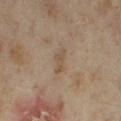Q: Was a biopsy performed?
A: no biopsy performed (imaged during a skin exam)
Q: Where on the body is the lesion?
A: the leg
Q: Lesion size?
A: ~3 mm (longest diameter)
Q: How was the tile lit?
A: cross-polarized illumination
Q: Patient demographics?
A: female, aged 33 to 37
Q: What kind of image is this?
A: 15 mm crop, total-body photography